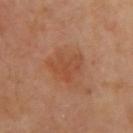Assessment:
No biopsy was performed on this lesion — it was imaged during a full skin examination and was not determined to be concerning.
Clinical summary:
The lesion is on the right upper arm. A 15 mm close-up tile from a total-body photography series done for melanoma screening. The lesion's longest dimension is about 4 mm.